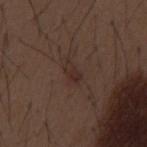Imaged during a routine full-body skin examination; the lesion was not biopsied and no histopathology is available.
Located on the mid back.
The lesion's longest dimension is about 3 mm.
A 15 mm crop from a total-body photograph taken for skin-cancer surveillance.
This is a white-light tile.
The subject is a male aged 48–52.